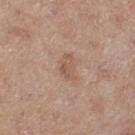biopsy status: catalogued during a skin exam; not biopsied
acquisition: ~15 mm crop, total-body skin-cancer survey
patient: female, about 45 years old
lighting: white-light
diameter: ≈3 mm
body site: the leg